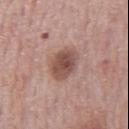The lesion was tiled from a total-body skin photograph and was not biopsied.
The subject is a male aged 73 to 77.
The lesion is on the mid back.
Longest diameter approximately 4 mm.
This is a white-light tile.
Cropped from a whole-body photographic skin survey; the tile spans about 15 mm.